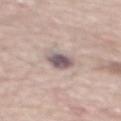{
  "biopsy_status": "not biopsied; imaged during a skin examination",
  "site": "mid back",
  "image": {
    "source": "total-body photography crop",
    "field_of_view_mm": 15
  },
  "patient": {
    "sex": "male",
    "age_approx": 70
  },
  "lighting": "white-light"
}